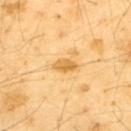follow-up: catalogued during a skin exam; not biopsied
image-analysis metrics: a lesion area of about 3.5 mm², an eccentricity of roughly 0.85, and two-axis asymmetry of about 0.15; an average lesion color of about L≈70 a*≈21 b*≈52 (CIELAB) and about 11 CIELAB-L* units darker than the surrounding skin; a border-irregularity rating of about 2/10 and a color-variation rating of about 2.5/10
anatomic site: the upper back
lighting: cross-polarized
acquisition: ~15 mm crop, total-body skin-cancer survey
subject: male, aged 63–67